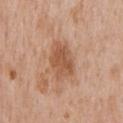Findings:
- workup: imaged on a skin check; not biopsied